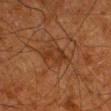Q: How was this image acquired?
A: 15 mm crop, total-body photography
Q: Who is the patient?
A: male, aged 78–82
Q: How was the tile lit?
A: cross-polarized illumination
Q: Lesion location?
A: the right lower leg